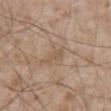Part of a total-body skin-imaging series; this lesion was reviewed on a skin check and was not flagged for biopsy. The tile uses white-light illumination. On the abdomen. The patient is a male approximately 55 years of age. A 15 mm close-up extracted from a 3D total-body photography capture.